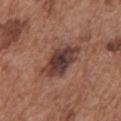Impression: The lesion was tiled from a total-body skin photograph and was not biopsied. Clinical summary: An algorithmic analysis of the crop reported a lesion area of about 14 mm² and a shape-asymmetry score of about 0.25 (0 = symmetric). The analysis additionally found about 12 CIELAB-L* units darker than the surrounding skin and a normalized border contrast of about 11. It also reported internal color variation of about 7 on a 0–10 scale and radial color variation of about 1.5. The lesion's longest dimension is about 6 mm. A male patient, aged around 75. The lesion is located on the front of the torso. A close-up tile cropped from a whole-body skin photograph, about 15 mm across. The tile uses white-light illumination.